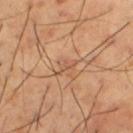- follow-up: imaged on a skin check; not biopsied
- patient: male, in their mid- to late 60s
- lesion size: ~3 mm (longest diameter)
- automated lesion analysis: a shape eccentricity near 0.9 and two-axis asymmetry of about 0.6; a border-irregularity index near 7.5/10; a classifier nevus-likeness of about 0/100 and a detector confidence of about 65 out of 100 that the crop contains a lesion
- imaging modality: ~15 mm crop, total-body skin-cancer survey
- tile lighting: cross-polarized
- location: the leg